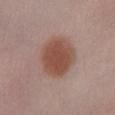Assessment: Imaged during a routine full-body skin examination; the lesion was not biopsied and no histopathology is available. Context: The patient is a female aged 63–67. This image is a 15 mm lesion crop taken from a total-body photograph. The total-body-photography lesion software estimated an average lesion color of about L≈49 a*≈21 b*≈26 (CIELAB), a lesion–skin lightness drop of about 11, and a normalized lesion–skin contrast near 9. Imaged with white-light lighting. Located on the right lower leg. The recorded lesion diameter is about 5 mm.